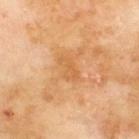  biopsy_status: not biopsied; imaged during a skin examination
  image:
    source: total-body photography crop
    field_of_view_mm: 15
  site: upper back
  automated_metrics:
    area_mm2_approx: 4.0
    shape_asymmetry: 0.4
  lesion_size:
    long_diameter_mm_approx: 3.0
  patient:
    sex: male
    age_approx: 70
  lighting: cross-polarized